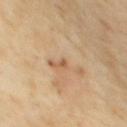Image and clinical context:
Imaged with cross-polarized lighting. Cropped from a whole-body photographic skin survey; the tile spans about 15 mm. A female subject aged around 70. On the upper back. Longest diameter approximately 1 mm. The lesion-visualizer software estimated an area of roughly 0.5 mm², an outline eccentricity of about 0.85 (0 = round, 1 = elongated), and a symmetry-axis asymmetry near 0.6. And it measured about 10 CIELAB-L* units darker than the surrounding skin and a normalized border contrast of about 7. The analysis additionally found a classifier nevus-likeness of about 0/100 and lesion-presence confidence of about 95/100.